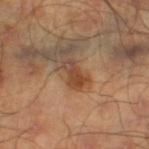Assessment: No biopsy was performed on this lesion — it was imaged during a full skin examination and was not determined to be concerning. Acquisition and patient details: A male subject about 45 years old. A 15 mm close-up tile from a total-body photography series done for melanoma screening. The lesion is on the leg.